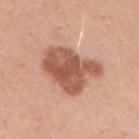Assessment:
The lesion was tiled from a total-body skin photograph and was not biopsied.
Background:
A 15 mm close-up tile from a total-body photography series done for melanoma screening. The lesion is on the right upper arm. A female subject aged 28 to 32.